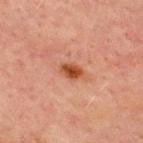Clinical impression: Imaged during a routine full-body skin examination; the lesion was not biopsied and no histopathology is available. Acquisition and patient details: The recorded lesion diameter is about 3 mm. A male subject, aged around 70. From the front of the torso. Cropped from a total-body skin-imaging series; the visible field is about 15 mm.